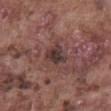  biopsy_status: not biopsied; imaged during a skin examination
  lesion_size:
    long_diameter_mm_approx: 3.0
  image:
    source: total-body photography crop
    field_of_view_mm: 15
  patient:
    sex: male
    age_approx: 75
  automated_metrics:
    area_mm2_approx: 5.5
    eccentricity: 0.55
    shape_asymmetry: 0.3
    vs_skin_darker_L: 10.0
    vs_skin_contrast_norm: 9.0
    border_irregularity_0_10: 2.5
    color_variation_0_10: 4.5
    peripheral_color_asymmetry: 1.5
  site: abdomen